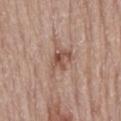Q: Is there a histopathology result?
A: total-body-photography surveillance lesion; no biopsy
Q: What are the patient's age and sex?
A: male, in their mid- to late 70s
Q: What kind of image is this?
A: total-body-photography crop, ~15 mm field of view
Q: What lighting was used for the tile?
A: white-light
Q: Lesion location?
A: the mid back
Q: What is the lesion's diameter?
A: ≈4 mm